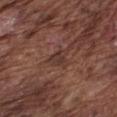Assessment:
Part of a total-body skin-imaging series; this lesion was reviewed on a skin check and was not flagged for biopsy.
Acquisition and patient details:
A male subject aged 73–77. Measured at roughly 3.5 mm in maximum diameter. The total-body-photography lesion software estimated a border-irregularity index near 4/10 and peripheral color asymmetry of about 0.5. The software also gave a detector confidence of about 95 out of 100 that the crop contains a lesion. A close-up tile cropped from a whole-body skin photograph, about 15 mm across. The lesion is located on the chest. The tile uses white-light illumination.